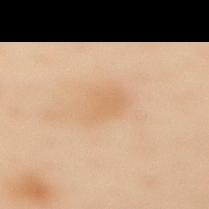Findings:
– notes — total-body-photography surveillance lesion; no biopsy
– diameter — ≈4 mm
– acquisition — 15 mm crop, total-body photography
– body site — the mid back
– illumination — cross-polarized illumination
– subject — female, about 60 years old